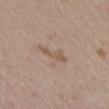This lesion was catalogued during total-body skin photography and was not selected for biopsy. The lesion is on the abdomen. A close-up tile cropped from a whole-body skin photograph, about 15 mm across. A female subject roughly 30 years of age.